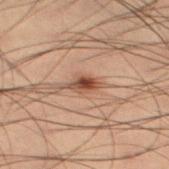<case>
  <patient>
    <sex>male</sex>
    <age_approx>55</age_approx>
  </patient>
  <image>
    <source>total-body photography crop</source>
    <field_of_view_mm>15</field_of_view_mm>
  </image>
  <site>leg</site>
</case>